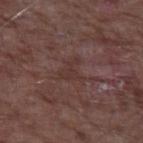Imaged during a routine full-body skin examination; the lesion was not biopsied and no histopathology is available. Cropped from a total-body skin-imaging series; the visible field is about 15 mm. Imaged with white-light lighting. The recorded lesion diameter is about 3 mm. An algorithmic analysis of the crop reported a lesion area of about 4.5 mm² and an eccentricity of roughly 0.45. The analysis additionally found a border-irregularity index near 7.5/10, internal color variation of about 1 on a 0–10 scale, and a peripheral color-asymmetry measure near 0.5. And it measured a classifier nevus-likeness of about 0/100 and a lesion-detection confidence of about 90/100. A male subject, aged 63–67. The lesion is on the left upper arm.